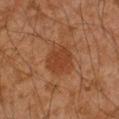Q: Was a biopsy performed?
A: imaged on a skin check; not biopsied
Q: Patient demographics?
A: male, aged around 45
Q: Lesion size?
A: ~4 mm (longest diameter)
Q: What is the anatomic site?
A: the arm
Q: What kind of image is this?
A: ~15 mm crop, total-body skin-cancer survey
Q: What lighting was used for the tile?
A: cross-polarized illumination